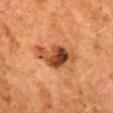Part of a total-body skin-imaging series; this lesion was reviewed on a skin check and was not flagged for biopsy. A female patient, in their 50s. Measured at roughly 6 mm in maximum diameter. Imaged with cross-polarized lighting. The lesion is on the mid back. A 15 mm close-up tile from a total-body photography series done for melanoma screening.